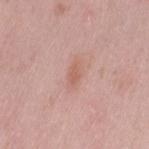follow-up = no biopsy performed (imaged during a skin exam); location = the left thigh; patient = female, roughly 40 years of age; acquisition = ~15 mm crop, total-body skin-cancer survey.